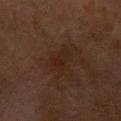workup=no biopsy performed (imaged during a skin exam); imaging modality=total-body-photography crop, ~15 mm field of view; lighting=cross-polarized illumination; patient=female, in their 70s; location=the left forearm.